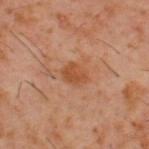{
  "biopsy_status": "not biopsied; imaged during a skin examination",
  "site": "upper back",
  "lighting": "cross-polarized",
  "patient": {
    "sex": "male",
    "age_approx": 60
  },
  "lesion_size": {
    "long_diameter_mm_approx": 3.0
  },
  "image": {
    "source": "total-body photography crop",
    "field_of_view_mm": 15
  }
}